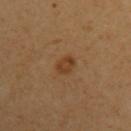Recorded during total-body skin imaging; not selected for excision or biopsy. Automated image analysis of the tile measured a lesion area of about 4.5 mm², an eccentricity of roughly 0.7, and a symmetry-axis asymmetry near 0.15. It also reported an average lesion color of about L≈39 a*≈19 b*≈34 (CIELAB) and a lesion–skin lightness drop of about 8. A region of skin cropped from a whole-body photographic capture, roughly 15 mm wide. Measured at roughly 3 mm in maximum diameter. The patient is a female aged 33–37. The lesion is located on the left upper arm.